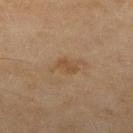The lesion was tiled from a total-body skin photograph and was not biopsied. A 15 mm crop from a total-body photograph taken for skin-cancer surveillance. A female subject, approximately 60 years of age. About 3.5 mm across. Located on the right lower leg.